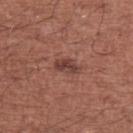workup: catalogued during a skin exam; not biopsied
acquisition: total-body-photography crop, ~15 mm field of view
location: the right upper arm
illumination: white-light
patient: male, approximately 45 years of age
TBP lesion metrics: an eccentricity of roughly 0.85; an average lesion color of about L≈41 a*≈24 b*≈23 (CIELAB), roughly 10 lightness units darker than nearby skin, and a lesion-to-skin contrast of about 8 (normalized; higher = more distinct); a border-irregularity rating of about 2.5/10, a within-lesion color-variation index near 3/10, and a peripheral color-asymmetry measure near 1; a detector confidence of about 100 out of 100 that the crop contains a lesion